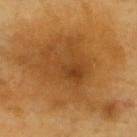No biopsy was performed on this lesion — it was imaged during a full skin examination and was not determined to be concerning. On the upper back. Automated image analysis of the tile measured a lesion area of about 95 mm² and an eccentricity of roughly 0.65. And it measured border irregularity of about 7 on a 0–10 scale, a color-variation rating of about 6/10, and peripheral color asymmetry of about 2. The software also gave an automated nevus-likeness rating near 65 out of 100. This image is a 15 mm lesion crop taken from a total-body photograph. The subject is a male about 60 years old.